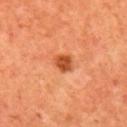Recorded during total-body skin imaging; not selected for excision or biopsy.
A region of skin cropped from a whole-body photographic capture, roughly 15 mm wide.
The lesion is on the mid back.
This is a cross-polarized tile.
The lesion-visualizer software estimated an outline eccentricity of about 0.55 (0 = round, 1 = elongated) and two-axis asymmetry of about 0.25. And it measured an average lesion color of about L≈49 a*≈31 b*≈42 (CIELAB) and a normalized border contrast of about 9.5. The analysis additionally found a border-irregularity index near 2/10 and a within-lesion color-variation index near 3/10.
A male patient, about 60 years old.
The lesion's longest dimension is about 2.5 mm.